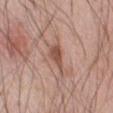Acquisition and patient details:
Imaged with white-light lighting. A male patient, about 60 years old. A close-up tile cropped from a whole-body skin photograph, about 15 mm across. The lesion is on the abdomen. The lesion's longest dimension is about 4.5 mm.